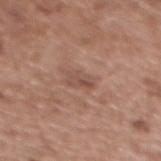| feature | finding |
|---|---|
| follow-up | catalogued during a skin exam; not biopsied |
| location | the mid back |
| patient | female, in their mid- to late 70s |
| acquisition | ~15 mm crop, total-body skin-cancer survey |
| diameter | about 3 mm |
| tile lighting | white-light |